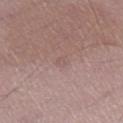Captured during whole-body skin photography for melanoma surveillance; the lesion was not biopsied. Captured under white-light illumination. The lesion is located on the right thigh. The recorded lesion diameter is about 1 mm. The patient is a male aged around 45. A roughly 15 mm field-of-view crop from a total-body skin photograph.